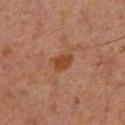Assessment:
Captured during whole-body skin photography for melanoma surveillance; the lesion was not biopsied.
Context:
From the left lower leg. Automated tile analysis of the lesion measured an average lesion color of about L≈41 a*≈24 b*≈33 (CIELAB), about 8 CIELAB-L* units darker than the surrounding skin, and a normalized border contrast of about 8. The software also gave a border-irregularity rating of about 2.5/10, internal color variation of about 2 on a 0–10 scale, and peripheral color asymmetry of about 0.5. The software also gave a nevus-likeness score of about 45/100 and a detector confidence of about 100 out of 100 that the crop contains a lesion. The lesion's longest dimension is about 3 mm. A roughly 15 mm field-of-view crop from a total-body skin photograph. Captured under cross-polarized illumination. A male subject aged 58 to 62.